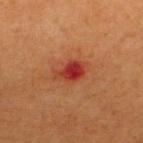| feature | finding |
|---|---|
| workup | total-body-photography surveillance lesion; no biopsy |